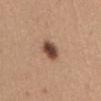Clinical impression: Captured during whole-body skin photography for melanoma surveillance; the lesion was not biopsied. Image and clinical context: Longest diameter approximately 3 mm. The tile uses white-light illumination. Automated tile analysis of the lesion measured an area of roughly 6 mm² and an outline eccentricity of about 0.5 (0 = round, 1 = elongated). The software also gave border irregularity of about 1.5 on a 0–10 scale and a within-lesion color-variation index near 4.5/10. And it measured an automated nevus-likeness rating near 95 out of 100 and a lesion-detection confidence of about 100/100. From the abdomen. A female subject, aged 28–32. This image is a 15 mm lesion crop taken from a total-body photograph.